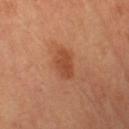Q: What are the patient's age and sex?
A: female, aged 58–62
Q: What did automated image analysis measure?
A: a footprint of about 7 mm², an outline eccentricity of about 0.8 (0 = round, 1 = elongated), and two-axis asymmetry of about 0.2; peripheral color asymmetry of about 1; an automated nevus-likeness rating near 90 out of 100 and a lesion-detection confidence of about 100/100
Q: What is the lesion's diameter?
A: ≈3.5 mm
Q: What is the anatomic site?
A: the left thigh
Q: What is the imaging modality?
A: total-body-photography crop, ~15 mm field of view
Q: Illumination type?
A: cross-polarized illumination